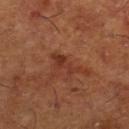notes: no biopsy performed (imaged during a skin exam); image: ~15 mm crop, total-body skin-cancer survey; illumination: cross-polarized illumination; patient: male, about 65 years old; body site: the right lower leg.